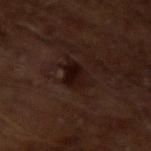A male patient, about 65 years old.
On the arm.
This image is a 15 mm lesion crop taken from a total-body photograph.
The recorded lesion diameter is about 4 mm.
Captured under cross-polarized illumination.